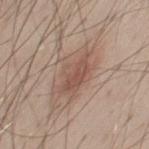<record>
  <biopsy_status>not biopsied; imaged during a skin examination</biopsy_status>
  <image>
    <source>total-body photography crop</source>
    <field_of_view_mm>15</field_of_view_mm>
  </image>
  <lesion_size>
    <long_diameter_mm_approx>6.5</long_diameter_mm_approx>
  </lesion_size>
  <patient>
    <sex>male</sex>
    <age_approx>45</age_approx>
  </patient>
  <site>chest</site>
  <lighting>white-light</lighting>
  <automated_metrics>
    <cielab_L>54</cielab_L>
    <cielab_a>18</cielab_a>
    <cielab_b>25</cielab_b>
    <vs_skin_darker_L>9.0</vs_skin_darker_L>
    <vs_skin_contrast_norm>6.5</vs_skin_contrast_norm>
    <border_irregularity_0_10>5.0</border_irregularity_0_10>
    <color_variation_0_10>5.0</color_variation_0_10>
    <peripheral_color_asymmetry>2.0</peripheral_color_asymmetry>
    <lesion_detection_confidence_0_100>100</lesion_detection_confidence_0_100>
  </automated_metrics>
</record>